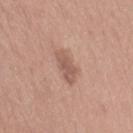The lesion was tiled from a total-body skin photograph and was not biopsied. The lesion is located on the arm. A 15 mm close-up tile from a total-body photography series done for melanoma screening. A female patient approximately 65 years of age.